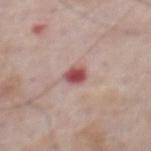Impression: Recorded during total-body skin imaging; not selected for excision or biopsy. Clinical summary: A region of skin cropped from a whole-body photographic capture, roughly 15 mm wide. On the abdomen. Imaged with white-light lighting. A male subject, aged approximately 75. The lesion-visualizer software estimated a footprint of about 4 mm², an eccentricity of roughly 0.65, and two-axis asymmetry of about 0.3. And it measured a lesion color around L≈51 a*≈29 b*≈22 in CIELAB, about 15 CIELAB-L* units darker than the surrounding skin, and a normalized border contrast of about 10.5. The analysis additionally found border irregularity of about 2.5 on a 0–10 scale, a within-lesion color-variation index near 3/10, and a peripheral color-asymmetry measure near 1. About 2.5 mm across.